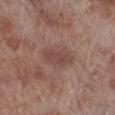Q: How was the tile lit?
A: white-light illumination
Q: Lesion size?
A: ≈4 mm
Q: Lesion location?
A: the left lower leg
Q: What are the patient's age and sex?
A: male, aged approximately 70
Q: What kind of image is this?
A: ~15 mm tile from a whole-body skin photo